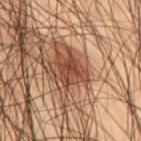Clinical impression:
No biopsy was performed on this lesion — it was imaged during a full skin examination and was not determined to be concerning.
Context:
A male subject aged 48–52. This image is a 15 mm lesion crop taken from a total-body photograph. The lesion-visualizer software estimated peripheral color asymmetry of about 1.5. The lesion is located on the lower back.